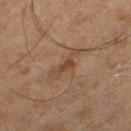<record>
<biopsy_status>not biopsied; imaged during a skin examination</biopsy_status>
<lesion_size>
  <long_diameter_mm_approx>4.5</long_diameter_mm_approx>
</lesion_size>
<image>
  <source>total-body photography crop</source>
  <field_of_view_mm>15</field_of_view_mm>
</image>
<site>left thigh</site>
<automated_metrics>
  <vs_skin_darker_L>6.0</vs_skin_darker_L>
  <vs_skin_contrast_norm>6.0</vs_skin_contrast_norm>
</automated_metrics>
<patient>
  <sex>male</sex>
  <age_approx>70</age_approx>
</patient>
</record>